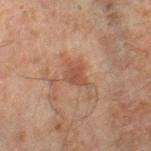Part of a total-body skin-imaging series; this lesion was reviewed on a skin check and was not flagged for biopsy.
A 15 mm close-up tile from a total-body photography series done for melanoma screening.
About 3 mm across.
A male subject, about 45 years old.
On the right lower leg.
Imaged with cross-polarized lighting.